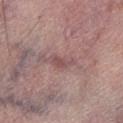Assessment: Recorded during total-body skin imaging; not selected for excision or biopsy. Acquisition and patient details: On the left lower leg. A lesion tile, about 15 mm wide, cut from a 3D total-body photograph. Approximately 3.5 mm at its widest. A male patient about 55 years old.